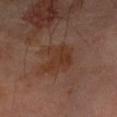Captured during whole-body skin photography for melanoma surveillance; the lesion was not biopsied.
Measured at roughly 4 mm in maximum diameter.
The lesion is located on the left forearm.
A region of skin cropped from a whole-body photographic capture, roughly 15 mm wide.
The tile uses cross-polarized illumination.
A male patient in their mid- to late 60s.